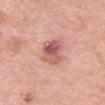Recorded during total-body skin imaging; not selected for excision or biopsy.
The subject is a female approximately 60 years of age.
The recorded lesion diameter is about 4 mm.
A lesion tile, about 15 mm wide, cut from a 3D total-body photograph.
The tile uses white-light illumination.
From the head or neck.
Automated tile analysis of the lesion measured a lesion area of about 9 mm² and a symmetry-axis asymmetry near 0.25. The software also gave a lesion color around L≈58 a*≈27 b*≈23 in CIELAB and a lesion–skin lightness drop of about 13. It also reported internal color variation of about 10 on a 0–10 scale and radial color variation of about 3. The software also gave a nevus-likeness score of about 30/100.